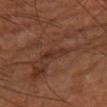{"biopsy_status": "not biopsied; imaged during a skin examination", "lighting": "cross-polarized", "lesion_size": {"long_diameter_mm_approx": 3.5}, "patient": {"age_approx": 65}, "automated_metrics": {"nevus_likeness_0_100": 0, "lesion_detection_confidence_0_100": 85}, "image": {"source": "total-body photography crop", "field_of_view_mm": 15}, "site": "right lower leg"}